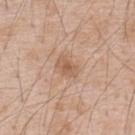Assessment:
Part of a total-body skin-imaging series; this lesion was reviewed on a skin check and was not flagged for biopsy.
Context:
About 3 mm across. A region of skin cropped from a whole-body photographic capture, roughly 15 mm wide. The subject is a male in their 50s. The total-body-photography lesion software estimated a footprint of about 4.5 mm², a shape eccentricity near 0.8, and a symmetry-axis asymmetry near 0.25. The analysis additionally found an automated nevus-likeness rating near 10 out of 100. Imaged with white-light lighting. From the back.